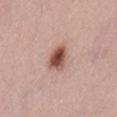Notes:
• follow-up · catalogued during a skin exam; not biopsied
• patient · male, aged around 25
• image-analysis metrics · an area of roughly 6.5 mm², an eccentricity of roughly 0.75, and a symmetry-axis asymmetry near 0.25
• image · ~15 mm crop, total-body skin-cancer survey
• tile lighting · white-light illumination
• site · the lower back